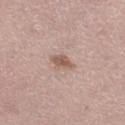Q: Was a biopsy performed?
A: no biopsy performed (imaged during a skin exam)
Q: Patient demographics?
A: female, aged approximately 45
Q: How was this image acquired?
A: ~15 mm crop, total-body skin-cancer survey
Q: Where on the body is the lesion?
A: the left lower leg